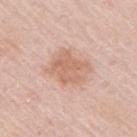Impression: Imaged during a routine full-body skin examination; the lesion was not biopsied and no histopathology is available. Image and clinical context: This is a white-light tile. A female subject approximately 65 years of age. On the right upper arm. A lesion tile, about 15 mm wide, cut from a 3D total-body photograph. The recorded lesion diameter is about 4.5 mm.